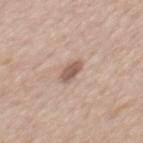Impression: The lesion was photographed on a routine skin check and not biopsied; there is no pathology result. Image and clinical context: A 15 mm crop from a total-body photograph taken for skin-cancer surveillance. A male subject, aged 63–67. The lesion is located on the back.